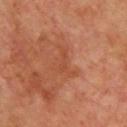Clinical impression:
This lesion was catalogued during total-body skin photography and was not selected for biopsy.
Context:
The total-body-photography lesion software estimated a mean CIELAB color near L≈47 a*≈27 b*≈34, a lesion–skin lightness drop of about 6, and a normalized lesion–skin contrast near 4.5. Longest diameter approximately 4.5 mm. A 15 mm close-up extracted from a 3D total-body photography capture. The patient is a male aged 63 to 67. On the chest.